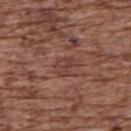follow-up: imaged on a skin check; not biopsied | site: the back | patient: female, aged 73 to 77 | lighting: white-light | imaging modality: ~15 mm tile from a whole-body skin photo.